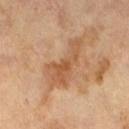Impression:
Recorded during total-body skin imaging; not selected for excision or biopsy.
Acquisition and patient details:
This is a cross-polarized tile. This image is a 15 mm lesion crop taken from a total-body photograph. A male patient about 65 years old. The recorded lesion diameter is about 6 mm. The total-body-photography lesion software estimated a lesion area of about 11 mm² and a shape-asymmetry score of about 0.55 (0 = symmetric). The analysis additionally found an average lesion color of about L≈53 a*≈21 b*≈36 (CIELAB), roughly 9 lightness units darker than nearby skin, and a normalized border contrast of about 6.5. The software also gave a nevus-likeness score of about 0/100 and lesion-presence confidence of about 100/100.